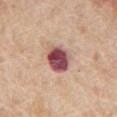  biopsy_status: not biopsied; imaged during a skin examination
  image:
    source: total-body photography crop
    field_of_view_mm: 15
  patient:
    sex: male
    age_approx: 65
  site: chest